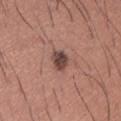workup = total-body-photography surveillance lesion; no biopsy | site = the lower back | patient = male, aged 43 to 47 | image source = ~15 mm crop, total-body skin-cancer survey.